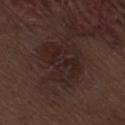Clinical impression: No biopsy was performed on this lesion — it was imaged during a full skin examination and was not determined to be concerning. Clinical summary: A region of skin cropped from a whole-body photographic capture, roughly 15 mm wide. Imaged with white-light lighting. The lesion is on the right thigh. Measured at roughly 6 mm in maximum diameter. A male patient aged 68 to 72.